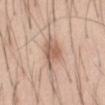| feature | finding |
|---|---|
| notes | imaged on a skin check; not biopsied |
| lesion diameter | ~4 mm (longest diameter) |
| automated lesion analysis | a lesion area of about 8 mm², an eccentricity of roughly 0.75, and two-axis asymmetry of about 0.3; border irregularity of about 3.5 on a 0–10 scale, internal color variation of about 3.5 on a 0–10 scale, and a peripheral color-asymmetry measure near 1 |
| lighting | white-light |
| acquisition | ~15 mm tile from a whole-body skin photo |
| patient | male, approximately 45 years of age |
| site | the abdomen |